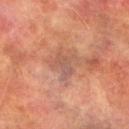Recorded during total-body skin imaging; not selected for excision or biopsy. The lesion is located on the left lower leg. A 15 mm close-up extracted from a 3D total-body photography capture. The patient is a male roughly 75 years of age.